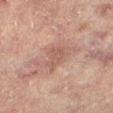Clinical impression: Recorded during total-body skin imaging; not selected for excision or biopsy.